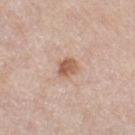Cropped from a total-body skin-imaging series; the visible field is about 15 mm.
The lesion is located on the left thigh.
The recorded lesion diameter is about 2.5 mm.
A male patient, in their mid-80s or older.
The tile uses white-light illumination.